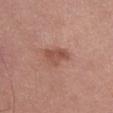The lesion was tiled from a total-body skin photograph and was not biopsied. From the abdomen. Approximately 3.5 mm at its widest. A region of skin cropped from a whole-body photographic capture, roughly 15 mm wide. The lesion-visualizer software estimated an outline eccentricity of about 0.8 (0 = round, 1 = elongated) and two-axis asymmetry of about 0.35. The software also gave a lesion color around L≈51 a*≈24 b*≈28 in CIELAB, about 9 CIELAB-L* units darker than the surrounding skin, and a lesion-to-skin contrast of about 7 (normalized; higher = more distinct). The software also gave a border-irregularity rating of about 3.5/10, a color-variation rating of about 3/10, and radial color variation of about 1. The software also gave a lesion-detection confidence of about 100/100. A female patient, about 55 years old.